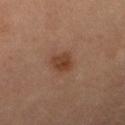follow-up=imaged on a skin check; not biopsied | body site=the right lower leg | image-analysis metrics=a border-irregularity index near 2/10, a within-lesion color-variation index near 2.5/10, and peripheral color asymmetry of about 1 | patient=female, in their mid- to late 50s | imaging modality=~15 mm tile from a whole-body skin photo | illumination=cross-polarized | lesion diameter=≈3 mm.